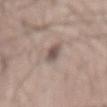* notes: imaged on a skin check; not biopsied
* lighting: white-light
* patient: male, aged 48 to 52
* lesion size: about 3.5 mm
* image: 15 mm crop, total-body photography
* body site: the mid back
* automated lesion analysis: a mean CIELAB color near L≈52 a*≈14 b*≈20 and a normalized lesion–skin contrast near 8.5; a lesion-detection confidence of about 75/100